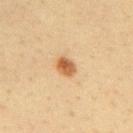Impression: Part of a total-body skin-imaging series; this lesion was reviewed on a skin check and was not flagged for biopsy. Clinical summary: Cropped from a total-body skin-imaging series; the visible field is about 15 mm. A male subject aged 38 to 42. The lesion is on the upper back.